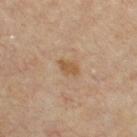Clinical impression: This lesion was catalogued during total-body skin photography and was not selected for biopsy. Clinical summary: A male subject, aged around 55. A region of skin cropped from a whole-body photographic capture, roughly 15 mm wide. The total-body-photography lesion software estimated a lesion area of about 3.5 mm² and an eccentricity of roughly 0.8. The analysis additionally found an average lesion color of about L≈50 a*≈16 b*≈33 (CIELAB), roughly 8 lightness units darker than nearby skin, and a normalized border contrast of about 7. It also reported a classifier nevus-likeness of about 0/100 and a lesion-detection confidence of about 100/100. On the chest.